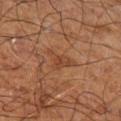From the left upper arm.
This is a cross-polarized tile.
Approximately 4 mm at its widest.
A 15 mm close-up extracted from a 3D total-body photography capture.
A male patient aged 68–72.